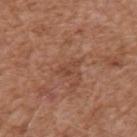Q: Was this lesion biopsied?
A: imaged on a skin check; not biopsied
Q: What are the patient's age and sex?
A: male, aged 73–77
Q: What is the anatomic site?
A: the upper back
Q: What did automated image analysis measure?
A: a footprint of about 3 mm² and a symmetry-axis asymmetry near 0.3; a lesion-to-skin contrast of about 5 (normalized; higher = more distinct); a border-irregularity rating of about 3.5/10, a within-lesion color-variation index near 1/10, and radial color variation of about 0.5
Q: How was the tile lit?
A: white-light illumination
Q: How was this image acquired?
A: ~15 mm crop, total-body skin-cancer survey